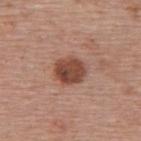Imaged during a routine full-body skin examination; the lesion was not biopsied and no histopathology is available. A female patient approximately 65 years of age. Automated tile analysis of the lesion measured an outline eccentricity of about 0.55 (0 = round, 1 = elongated). The analysis additionally found a lesion color around L≈46 a*≈23 b*≈28 in CIELAB, roughly 14 lightness units darker than nearby skin, and a normalized border contrast of about 10. The lesion is on the upper back. Cropped from a total-body skin-imaging series; the visible field is about 15 mm.